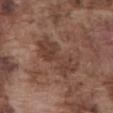Findings:
* follow-up · no biopsy performed (imaged during a skin exam)
* site · the abdomen
* illumination · white-light illumination
* subject · male, about 75 years old
* image · ~15 mm crop, total-body skin-cancer survey